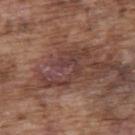  biopsy_status: not biopsied; imaged during a skin examination
  image:
    source: total-body photography crop
    field_of_view_mm: 15
  lesion_size:
    long_diameter_mm_approx: 6.5
  automated_metrics:
    cielab_L: 39
    cielab_a: 20
    cielab_b: 22
    vs_skin_contrast_norm: 7.5
    color_variation_0_10: 2.5
    peripheral_color_asymmetry: 0.5
  site: upper back
  patient:
    sex: male
    age_approx: 75
  lighting: white-light